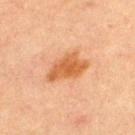This lesion was catalogued during total-body skin photography and was not selected for biopsy. The lesion is located on the upper back. A male patient, aged approximately 60. Automated image analysis of the tile measured a footprint of about 11 mm², an outline eccentricity of about 0.8 (0 = round, 1 = elongated), and a symmetry-axis asymmetry near 0.3. And it measured a color-variation rating of about 2.5/10 and radial color variation of about 1. The analysis additionally found a classifier nevus-likeness of about 90/100. Measured at roughly 5 mm in maximum diameter. Cropped from a total-body skin-imaging series; the visible field is about 15 mm. Captured under cross-polarized illumination.